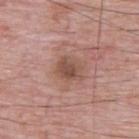Findings:
- subject: male, aged 63–67
- site: the upper back
- image: total-body-photography crop, ~15 mm field of view